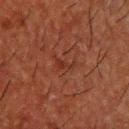Clinical impression: No biopsy was performed on this lesion — it was imaged during a full skin examination and was not determined to be concerning. Background: A 15 mm crop from a total-body photograph taken for skin-cancer surveillance. The tile uses cross-polarized illumination. Located on the upper back. The subject is a male approximately 50 years of age.